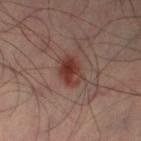The lesion was photographed on a routine skin check and not biopsied; there is no pathology result.
A lesion tile, about 15 mm wide, cut from a 3D total-body photograph.
Approximately 3.5 mm at its widest.
The patient is a male aged around 40.
Automated tile analysis of the lesion measured a mean CIELAB color near L≈36 a*≈23 b*≈24, roughly 10 lightness units darker than nearby skin, and a lesion-to-skin contrast of about 9 (normalized; higher = more distinct). The analysis additionally found a nevus-likeness score of about 100/100 and a detector confidence of about 100 out of 100 that the crop contains a lesion.
Captured under cross-polarized illumination.
On the left lower leg.